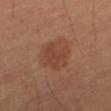{
  "biopsy_status": "not biopsied; imaged during a skin examination",
  "patient": {
    "sex": "male",
    "age_approx": 60
  },
  "lighting": "cross-polarized",
  "site": "right thigh",
  "lesion_size": {
    "long_diameter_mm_approx": 5.0
  },
  "automated_metrics": {
    "nevus_likeness_0_100": 60,
    "lesion_detection_confidence_0_100": 100
  },
  "image": {
    "source": "total-body photography crop",
    "field_of_view_mm": 15
  }
}